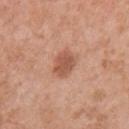  biopsy_status: not biopsied; imaged during a skin examination
  patient:
    sex: female
    age_approx: 50
  site: right upper arm
  image:
    source: total-body photography crop
    field_of_view_mm: 15
  automated_metrics:
    area_mm2_approx: 6.0
    eccentricity: 0.65
    shape_asymmetry: 0.2
    border_irregularity_0_10: 2.0
    color_variation_0_10: 2.0
    peripheral_color_asymmetry: 0.5
  lighting: white-light